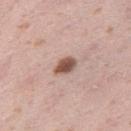{"biopsy_status": "not biopsied; imaged during a skin examination", "image": {"source": "total-body photography crop", "field_of_view_mm": 15}, "automated_metrics": {"area_mm2_approx": 4.5, "eccentricity": 0.7, "shape_asymmetry": 0.2, "cielab_L": 53, "cielab_a": 21, "cielab_b": 25, "vs_skin_darker_L": 16.0, "vs_skin_contrast_norm": 11.0, "nevus_likeness_0_100": 95}, "patient": {"sex": "female", "age_approx": 40}, "site": "right thigh", "lesion_size": {"long_diameter_mm_approx": 3.0}, "lighting": "white-light"}